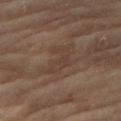notes: imaged on a skin check; not biopsied | automated lesion analysis: a lesion area of about 6.5 mm² and a shape-asymmetry score of about 0.65 (0 = symmetric); border irregularity of about 7.5 on a 0–10 scale and a color-variation rating of about 1/10; a nevus-likeness score of about 0/100 and lesion-presence confidence of about 75/100 | subject: female, in their 70s | image: total-body-photography crop, ~15 mm field of view | lesion size: ~4 mm (longest diameter) | tile lighting: cross-polarized illumination | location: the right thigh.